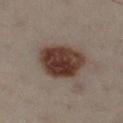{"biopsy_status": "not biopsied; imaged during a skin examination", "lighting": "cross-polarized", "patient": {"sex": "male", "age_approx": 50}, "lesion_size": {"long_diameter_mm_approx": 5.5}, "image": {"source": "total-body photography crop", "field_of_view_mm": 15}, "site": "left lower leg", "automated_metrics": {"vs_skin_darker_L": 14.0, "vs_skin_contrast_norm": 13.5, "border_irregularity_0_10": 1.5, "color_variation_0_10": 4.5, "peripheral_color_asymmetry": 1.5, "nevus_likeness_0_100": 100}}